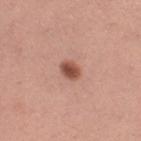biopsy_status: not biopsied; imaged during a skin examination
image:
  source: total-body photography crop
  field_of_view_mm: 15
patient:
  sex: female
  age_approx: 30
site: leg
lighting: white-light